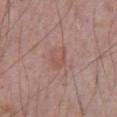workup: no biopsy performed (imaged during a skin exam); body site: the abdomen; lighting: white-light illumination; imaging modality: 15 mm crop, total-body photography; subject: male, aged 68 to 72.